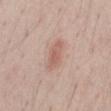Recorded during total-body skin imaging; not selected for excision or biopsy.
The lesion-visualizer software estimated a lesion area of about 6 mm² and two-axis asymmetry of about 0.2. The analysis additionally found a mean CIELAB color near L≈60 a*≈21 b*≈25, roughly 9 lightness units darker than nearby skin, and a normalized border contrast of about 6. And it measured border irregularity of about 2.5 on a 0–10 scale, internal color variation of about 2 on a 0–10 scale, and a peripheral color-asymmetry measure near 0.5. The software also gave an automated nevus-likeness rating near 25 out of 100 and a detector confidence of about 100 out of 100 that the crop contains a lesion.
The subject is a male roughly 60 years of age.
The lesion is on the abdomen.
Imaged with white-light lighting.
A 15 mm close-up extracted from a 3D total-body photography capture.